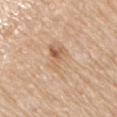follow-up: imaged on a skin check; not biopsied
diameter: ~5 mm (longest diameter)
automated metrics: a footprint of about 12 mm², an eccentricity of roughly 0.8, and a shape-asymmetry score of about 0.2 (0 = symmetric); a border-irregularity index near 3/10 and internal color variation of about 9 on a 0–10 scale; a classifier nevus-likeness of about 0/100
anatomic site: the left arm
image source: total-body-photography crop, ~15 mm field of view
patient: female, aged around 65